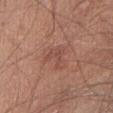Findings:
* image source: 15 mm crop, total-body photography
* body site: the abdomen
* subject: female, aged around 55